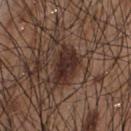Imaged during a routine full-body skin examination; the lesion was not biopsied and no histopathology is available. A male subject, in their mid-50s. The lesion is located on the chest. This image is a 15 mm lesion crop taken from a total-body photograph. Approximately 4.5 mm at its widest. Imaged with white-light lighting. The total-body-photography lesion software estimated a lesion area of about 8.5 mm², an outline eccentricity of about 0.85 (0 = round, 1 = elongated), and a symmetry-axis asymmetry near 0.25. The analysis additionally found an automated nevus-likeness rating near 80 out of 100.